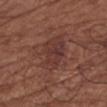Clinical summary: Measured at roughly 4.5 mm in maximum diameter. A 15 mm crop from a total-body photograph taken for skin-cancer surveillance. Automated image analysis of the tile measured a lesion area of about 15 mm², a shape eccentricity near 0.45, and a shape-asymmetry score of about 0.2 (0 = symmetric). The tile uses white-light illumination. A female patient, aged 78–82. From the arm.